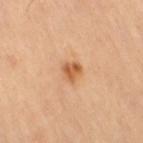Assessment: No biopsy was performed on this lesion — it was imaged during a full skin examination and was not determined to be concerning.